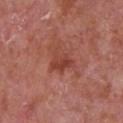Context:
This is a white-light tile. A male patient, in their mid- to late 60s. A 15 mm close-up tile from a total-body photography series done for melanoma screening. On the chest. The recorded lesion diameter is about 3.5 mm.